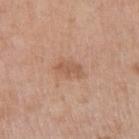workup = imaged on a skin check; not biopsied
patient = male, approximately 80 years of age
automated metrics = a border-irregularity rating of about 3.5/10, a within-lesion color-variation index near 1.5/10, and radial color variation of about 0.5
tile lighting = white-light
image = ~15 mm crop, total-body skin-cancer survey
location = the right upper arm